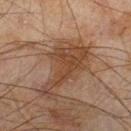Clinical impression:
Part of a total-body skin-imaging series; this lesion was reviewed on a skin check and was not flagged for biopsy.
Image and clinical context:
The lesion-visualizer software estimated a lesion area of about 15 mm², an outline eccentricity of about 0.9 (0 = round, 1 = elongated), and a symmetry-axis asymmetry near 0.65. And it measured a lesion color around L≈34 a*≈17 b*≈25 in CIELAB, a lesion–skin lightness drop of about 8, and a normalized lesion–skin contrast near 7.5. From the right lower leg. A male subject approximately 45 years of age. A region of skin cropped from a whole-body photographic capture, roughly 15 mm wide. Longest diameter approximately 7.5 mm. Imaged with cross-polarized lighting.